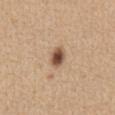| key | value |
|---|---|
| follow-up | imaged on a skin check; not biopsied |
| image-analysis metrics | an average lesion color of about L≈52 a*≈18 b*≈30 (CIELAB), a lesion–skin lightness drop of about 16, and a normalized lesion–skin contrast near 10.5; a border-irregularity rating of about 1/10 and a color-variation rating of about 5.5/10; a classifier nevus-likeness of about 100/100 and lesion-presence confidence of about 100/100 |
| body site | the front of the torso |
| acquisition | 15 mm crop, total-body photography |
| illumination | white-light illumination |
| patient | female, aged 58–62 |